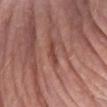The lesion was tiled from a total-body skin photograph and was not biopsied.
A female patient, aged approximately 70.
Captured under white-light illumination.
Located on the right forearm.
A 15 mm close-up tile from a total-body photography series done for melanoma screening.
About 3 mm across.